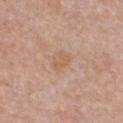Clinical impression: Imaged during a routine full-body skin examination; the lesion was not biopsied and no histopathology is available. Image and clinical context: Automated tile analysis of the lesion measured a footprint of about 4 mm², an outline eccentricity of about 0.7 (0 = round, 1 = elongated), and a shape-asymmetry score of about 0.25 (0 = symmetric). And it measured a border-irregularity rating of about 2.5/10, internal color variation of about 2 on a 0–10 scale, and radial color variation of about 1. And it measured a detector confidence of about 100 out of 100 that the crop contains a lesion. Measured at roughly 2.5 mm in maximum diameter. A female subject, aged 38–42. The tile uses white-light illumination. The lesion is on the chest. A lesion tile, about 15 mm wide, cut from a 3D total-body photograph.